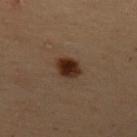Impression: The lesion was photographed on a routine skin check and not biopsied; there is no pathology result. Clinical summary: Located on the back. A female subject, aged 48 to 52. A roughly 15 mm field-of-view crop from a total-body skin photograph. This is a cross-polarized tile. The lesion's longest dimension is about 3.5 mm.